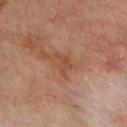| key | value |
|---|---|
| follow-up | imaged on a skin check; not biopsied |
| subject | male, about 70 years old |
| image source | ~15 mm tile from a whole-body skin photo |
| location | the chest |